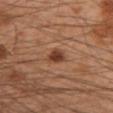| feature | finding |
|---|---|
| workup | no biopsy performed (imaged during a skin exam) |
| patient | male, about 50 years old |
| site | the left forearm |
| acquisition | ~15 mm crop, total-body skin-cancer survey |
| size | ~2.5 mm (longest diameter) |
| tile lighting | cross-polarized |